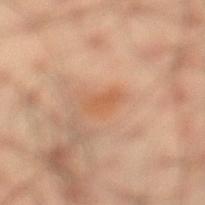{
  "biopsy_status": "not biopsied; imaged during a skin examination",
  "lighting": "cross-polarized",
  "lesion_size": {
    "long_diameter_mm_approx": 3.0
  },
  "site": "left lower leg",
  "patient": {
    "sex": "male",
    "age_approx": 35
  },
  "image": {
    "source": "total-body photography crop",
    "field_of_view_mm": 15
  }
}